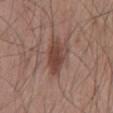Clinical summary: From the back. A male patient, about 55 years old. A close-up tile cropped from a whole-body skin photograph, about 15 mm across. Captured under white-light illumination.